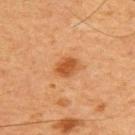biopsy_status: not biopsied; imaged during a skin examination
automated_metrics:
  shape_asymmetry: 0.25
  nevus_likeness_0_100: 90
patient:
  sex: male
  age_approx: 60
site: chest
lesion_size:
  long_diameter_mm_approx: 3.0
lighting: cross-polarized
image:
  source: total-body photography crop
  field_of_view_mm: 15